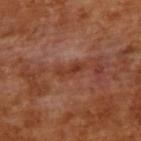| feature | finding |
|---|---|
| notes | total-body-photography surveillance lesion; no biopsy |
| illumination | cross-polarized illumination |
| subject | male, in their mid- to late 60s |
| imaging modality | ~15 mm tile from a whole-body skin photo |
| diameter | ≈3.5 mm |